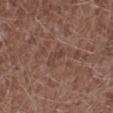Q: Is there a histopathology result?
A: no biopsy performed (imaged during a skin exam)
Q: Automated lesion metrics?
A: an area of roughly 2.5 mm², an outline eccentricity of about 0.9 (0 = round, 1 = elongated), and two-axis asymmetry of about 0.6; a lesion color around L≈41 a*≈19 b*≈24 in CIELAB and a normalized lesion–skin contrast near 5; a border-irregularity index near 7/10, internal color variation of about 0 on a 0–10 scale, and radial color variation of about 0; a nevus-likeness score of about 0/100 and lesion-presence confidence of about 95/100
Q: How was the tile lit?
A: white-light illumination
Q: How large is the lesion?
A: ≈2.5 mm
Q: What is the anatomic site?
A: the left lower leg
Q: Patient demographics?
A: male, approximately 45 years of age
Q: What kind of image is this?
A: total-body-photography crop, ~15 mm field of view